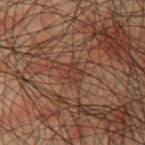Clinical impression: This lesion was catalogued during total-body skin photography and was not selected for biopsy. Background: Approximately 3 mm at its widest. A male subject aged 48 to 52. Imaged with cross-polarized lighting. A 15 mm close-up tile from a total-body photography series done for melanoma screening. From the chest.